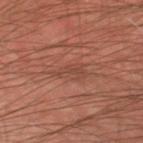Recorded during total-body skin imaging; not selected for excision or biopsy. A male patient approximately 45 years of age. The lesion's longest dimension is about 4 mm. Automated image analysis of the tile measured an outline eccentricity of about 0.9 (0 = round, 1 = elongated) and a shape-asymmetry score of about 0.45 (0 = symmetric). It also reported an average lesion color of about L≈42 a*≈22 b*≈27 (CIELAB), a lesion–skin lightness drop of about 6, and a normalized lesion–skin contrast near 4.5. The analysis additionally found a border-irregularity index near 5.5/10, internal color variation of about 1 on a 0–10 scale, and radial color variation of about 0.5. Imaged with cross-polarized lighting. A 15 mm close-up tile from a total-body photography series done for melanoma screening. The lesion is on the left thigh.